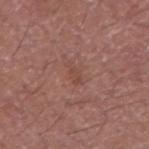{
  "biopsy_status": "not biopsied; imaged during a skin examination",
  "image": {
    "source": "total-body photography crop",
    "field_of_view_mm": 15
  },
  "site": "right upper arm",
  "lighting": "white-light",
  "lesion_size": {
    "long_diameter_mm_approx": 2.5
  },
  "patient": {
    "sex": "male",
    "age_approx": 70
  }
}